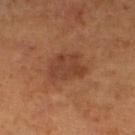Clinical impression:
Imaged during a routine full-body skin examination; the lesion was not biopsied and no histopathology is available.
Context:
The patient is a female aged approximately 65. The tile uses cross-polarized illumination. About 4.5 mm across. A lesion tile, about 15 mm wide, cut from a 3D total-body photograph. Automated image analysis of the tile measured an area of roughly 11 mm² and a symmetry-axis asymmetry near 0.25. It also reported a mean CIELAB color near L≈40 a*≈22 b*≈30, a lesion–skin lightness drop of about 8, and a lesion-to-skin contrast of about 6.5 (normalized; higher = more distinct). It also reported a within-lesion color-variation index near 2/10 and peripheral color asymmetry of about 0.5. The lesion is on the left lower leg.